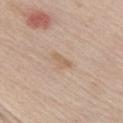Captured during whole-body skin photography for melanoma surveillance; the lesion was not biopsied. The subject is a male aged around 65. A 15 mm close-up tile from a total-body photography series done for melanoma screening. Measured at roughly 2.5 mm in maximum diameter. On the chest.